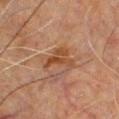Findings:
* biopsy status · no biopsy performed (imaged during a skin exam)
* location · the chest
* image · total-body-photography crop, ~15 mm field of view
* lesion diameter · about 4 mm
* illumination · cross-polarized
* subject · male, aged approximately 50
* TBP lesion metrics · an outline eccentricity of about 0.65 (0 = round, 1 = elongated); an average lesion color of about L≈37 a*≈18 b*≈28 (CIELAB), roughly 7 lightness units darker than nearby skin, and a normalized lesion–skin contrast near 7.5; a color-variation rating of about 3.5/10 and peripheral color asymmetry of about 1